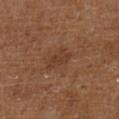{
  "biopsy_status": "not biopsied; imaged during a skin examination",
  "patient": {
    "sex": "male",
    "age_approx": 60
  },
  "automated_metrics": {
    "area_mm2_approx": 4.5,
    "eccentricity": 0.75,
    "shape_asymmetry": 0.25,
    "cielab_L": 36,
    "cielab_a": 20,
    "cielab_b": 29,
    "vs_skin_darker_L": 6.0,
    "vs_skin_contrast_norm": 5.5,
    "lesion_detection_confidence_0_100": 100
  },
  "lighting": "cross-polarized",
  "lesion_size": {
    "long_diameter_mm_approx": 3.0
  },
  "site": "leg",
  "image": {
    "source": "total-body photography crop",
    "field_of_view_mm": 15
  }
}